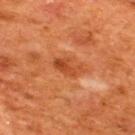{
  "biopsy_status": "not biopsied; imaged during a skin examination",
  "image": {
    "source": "total-body photography crop",
    "field_of_view_mm": 15
  },
  "lighting": "cross-polarized",
  "lesion_size": {
    "long_diameter_mm_approx": 3.5
  },
  "patient": {
    "sex": "male",
    "age_approx": 65
  },
  "site": "upper back"
}